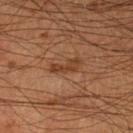Part of a total-body skin-imaging series; this lesion was reviewed on a skin check and was not flagged for biopsy.
The patient is a male in their mid-50s.
On the left lower leg.
This is a cross-polarized tile.
A 15 mm crop from a total-body photograph taken for skin-cancer surveillance.
Longest diameter approximately 3.5 mm.